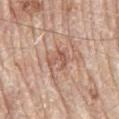A male patient roughly 80 years of age.
From the mid back.
This is a white-light tile.
A close-up tile cropped from a whole-body skin photograph, about 15 mm across.
Automated tile analysis of the lesion measured an area of roughly 3.5 mm² and two-axis asymmetry of about 0.55. And it measured a within-lesion color-variation index near 0.5/10 and peripheral color asymmetry of about 0. It also reported a classifier nevus-likeness of about 0/100 and a lesion-detection confidence of about 95/100.
The recorded lesion diameter is about 2.5 mm.